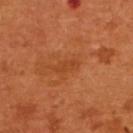– follow-up · catalogued during a skin exam; not biopsied
– size · ~3 mm (longest diameter)
– subject · female, aged approximately 55
– site · the upper back
– automated lesion analysis · a border-irregularity rating of about 4/10, a color-variation rating of about 1.5/10, and radial color variation of about 0.5; an automated nevus-likeness rating near 0 out of 100
– image source · ~15 mm tile from a whole-body skin photo
– illumination · cross-polarized illumination